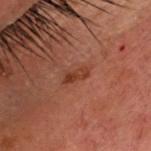Recorded during total-body skin imaging; not selected for excision or biopsy.
Cropped from a total-body skin-imaging series; the visible field is about 15 mm.
Located on the head or neck.
A male patient, about 40 years old.